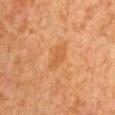The lesion is located on the chest. The subject is a male in their 80s. A 15 mm close-up extracted from a 3D total-body photography capture.